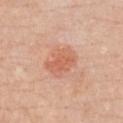Part of a total-body skin-imaging series; this lesion was reviewed on a skin check and was not flagged for biopsy. A region of skin cropped from a whole-body photographic capture, roughly 15 mm wide. The lesion is located on the chest. An algorithmic analysis of the crop reported an area of roughly 11 mm² and an outline eccentricity of about 0.6 (0 = round, 1 = elongated). A female subject in their 40s. Imaged with white-light lighting. The recorded lesion diameter is about 4 mm.